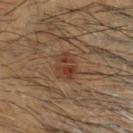Clinical impression: Recorded during total-body skin imaging; not selected for excision or biopsy. Context: The total-body-photography lesion software estimated roughly 7 lightness units darker than nearby skin and a normalized lesion–skin contrast near 6.5. The analysis additionally found border irregularity of about 3.5 on a 0–10 scale and internal color variation of about 4.5 on a 0–10 scale. And it measured an automated nevus-likeness rating near 15 out of 100 and a detector confidence of about 100 out of 100 that the crop contains a lesion. Longest diameter approximately 3 mm. A male patient aged around 60. A 15 mm close-up tile from a total-body photography series done for melanoma screening.